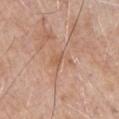follow-up: total-body-photography surveillance lesion; no biopsy
image: total-body-photography crop, ~15 mm field of view
illumination: white-light illumination
size: about 3 mm
location: the front of the torso
patient: male, aged around 80